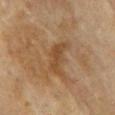{"lesion_size": {"long_diameter_mm_approx": 4.0}, "lighting": "cross-polarized", "patient": {"sex": "female", "age_approx": 60}, "automated_metrics": {"eccentricity": 0.9, "border_irregularity_0_10": 5.0, "peripheral_color_asymmetry": 0.5, "nevus_likeness_0_100": 0, "lesion_detection_confidence_0_100": 100}, "image": {"source": "total-body photography crop", "field_of_view_mm": 15}, "site": "chest"}